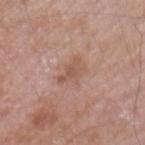Notes:
• workup · total-body-photography surveillance lesion; no biopsy
• location · the left upper arm
• lesion size · ≈3.5 mm
• TBP lesion metrics · a nevus-likeness score of about 0/100 and lesion-presence confidence of about 100/100
• image source · 15 mm crop, total-body photography
• lighting · white-light illumination
• patient · male, approximately 60 years of age